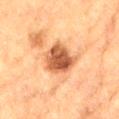Part of a total-body skin-imaging series; this lesion was reviewed on a skin check and was not flagged for biopsy.
On the lower back.
The lesion-visualizer software estimated a footprint of about 11 mm², an outline eccentricity of about 0.65 (0 = round, 1 = elongated), and a shape-asymmetry score of about 0.25 (0 = symmetric). The software also gave a lesion color around L≈51 a*≈25 b*≈36 in CIELAB, about 17 CIELAB-L* units darker than the surrounding skin, and a normalized lesion–skin contrast near 11. The analysis additionally found an automated nevus-likeness rating near 25 out of 100 and lesion-presence confidence of about 100/100.
This image is a 15 mm lesion crop taken from a total-body photograph.
Imaged with cross-polarized lighting.
A male subject aged around 85 or older.
About 4.5 mm across.